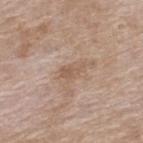<tbp_lesion>
  <biopsy_status>not biopsied; imaged during a skin examination</biopsy_status>
  <patient>
    <sex>female</sex>
    <age_approx>75</age_approx>
  </patient>
  <image>
    <source>total-body photography crop</source>
    <field_of_view_mm>15</field_of_view_mm>
  </image>
  <site>upper back</site>
  <lighting>white-light</lighting>
  <lesion_size>
    <long_diameter_mm_approx>3.0</long_diameter_mm_approx>
  </lesion_size>
  <automated_metrics>
    <cielab_L>56</cielab_L>
    <cielab_a>17</cielab_a>
    <cielab_b>29</cielab_b>
    <vs_skin_darker_L>8.0</vs_skin_darker_L>
    <nevus_likeness_0_100>0</nevus_likeness_0_100>
    <lesion_detection_confidence_0_100>100</lesion_detection_confidence_0_100>
  </automated_metrics>
</tbp_lesion>